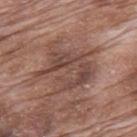notes: imaged on a skin check; not biopsied
acquisition: ~15 mm crop, total-body skin-cancer survey
patient: male, aged 68 to 72
location: the mid back